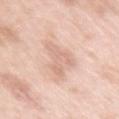Assessment:
Imaged during a routine full-body skin examination; the lesion was not biopsied and no histopathology is available.
Image and clinical context:
A region of skin cropped from a whole-body photographic capture, roughly 15 mm wide. The tile uses white-light illumination. The lesion is located on the right upper arm. The recorded lesion diameter is about 5 mm. Automated image analysis of the tile measured an area of roughly 8.5 mm². The software also gave an average lesion color of about L≈71 a*≈20 b*≈28 (CIELAB), roughly 8 lightness units darker than nearby skin, and a lesion-to-skin contrast of about 5 (normalized; higher = more distinct). It also reported border irregularity of about 7 on a 0–10 scale and internal color variation of about 2 on a 0–10 scale. A female patient, about 40 years old.